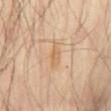The lesion was tiled from a total-body skin photograph and was not biopsied. A region of skin cropped from a whole-body photographic capture, roughly 15 mm wide. The patient is a male in their 70s. From the front of the torso.